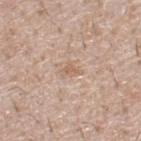This lesion was catalogued during total-body skin photography and was not selected for biopsy. Approximately 2.5 mm at its widest. The lesion is on the upper back. The patient is a male roughly 55 years of age. This is a white-light tile. A roughly 15 mm field-of-view crop from a total-body skin photograph.